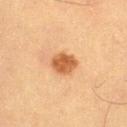Impression: This lesion was catalogued during total-body skin photography and was not selected for biopsy. Background: A male subject, aged 58–62. Longest diameter approximately 3.5 mm. The lesion is on the left thigh. A close-up tile cropped from a whole-body skin photograph, about 15 mm across.